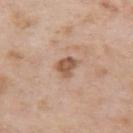| feature | finding |
|---|---|
| workup | imaged on a skin check; not biopsied |
| illumination | white-light |
| lesion diameter | ≈2.5 mm |
| site | the arm |
| image | ~15 mm tile from a whole-body skin photo |
| image-analysis metrics | a lesion color around L≈55 a*≈19 b*≈31 in CIELAB, about 12 CIELAB-L* units darker than the surrounding skin, and a normalized lesion–skin contrast near 8.5; border irregularity of about 3 on a 0–10 scale, a color-variation rating of about 2/10, and a peripheral color-asymmetry measure near 0.5; a classifier nevus-likeness of about 25/100 and a detector confidence of about 100 out of 100 that the crop contains a lesion |
| patient | male, aged 58–62 |